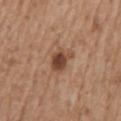This is a white-light tile. Approximately 3 mm at its widest. The lesion is on the mid back. The patient is a male aged around 70. A 15 mm crop from a total-body photograph taken for skin-cancer surveillance.